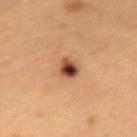<tbp_lesion>
<biopsy_status>not biopsied; imaged during a skin examination</biopsy_status>
<lesion_size>
  <long_diameter_mm_approx>3.0</long_diameter_mm_approx>
</lesion_size>
<automated_metrics>
  <cielab_L>46</cielab_L>
  <cielab_a>23</cielab_a>
  <cielab_b>34</cielab_b>
  <vs_skin_darker_L>19.0</vs_skin_darker_L>
  <vs_skin_contrast_norm>13.0</vs_skin_contrast_norm>
  <nevus_likeness_0_100>100</nevus_likeness_0_100>
  <lesion_detection_confidence_0_100>100</lesion_detection_confidence_0_100>
</automated_metrics>
<lighting>cross-polarized</lighting>
<image>
  <source>total-body photography crop</source>
  <field_of_view_mm>15</field_of_view_mm>
</image>
<site>left upper arm</site>
<patient>
  <sex>male</sex>
  <age_approx>60</age_approx>
</patient>
</tbp_lesion>